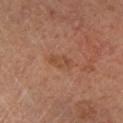Imaged during a routine full-body skin examination; the lesion was not biopsied and no histopathology is available. A male subject, aged around 65. Located on the leg. A 15 mm crop from a total-body photograph taken for skin-cancer surveillance.